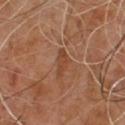Recorded during total-body skin imaging; not selected for excision or biopsy. Measured at roughly 3.5 mm in maximum diameter. Captured under cross-polarized illumination. A male patient in their mid-60s. A 15 mm crop from a total-body photograph taken for skin-cancer surveillance.